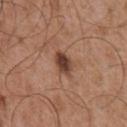follow-up: imaged on a skin check; not biopsied
anatomic site: the chest
imaging modality: total-body-photography crop, ~15 mm field of view
subject: male, in their mid-50s